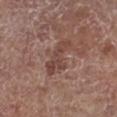| field | value |
|---|---|
| biopsy status | no biopsy performed (imaged during a skin exam) |
| acquisition | 15 mm crop, total-body photography |
| diameter | about 5 mm |
| subject | male, aged around 70 |
| illumination | white-light |
| image-analysis metrics | a shape eccentricity near 0.9 and a symmetry-axis asymmetry near 0.5; a detector confidence of about 100 out of 100 that the crop contains a lesion |
| anatomic site | the right lower leg |